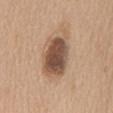Q: Is there a histopathology result?
A: catalogued during a skin exam; not biopsied
Q: How was the tile lit?
A: white-light
Q: What are the patient's age and sex?
A: female, aged 68–72
Q: What did automated image analysis measure?
A: an eccentricity of roughly 0.85 and a symmetry-axis asymmetry near 0.25
Q: How large is the lesion?
A: ≈7 mm
Q: Lesion location?
A: the mid back
Q: How was this image acquired?
A: ~15 mm crop, total-body skin-cancer survey